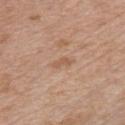{"biopsy_status": "not biopsied; imaged during a skin examination", "lesion_size": {"long_diameter_mm_approx": 2.5}, "automated_metrics": {"cielab_L": 57, "cielab_a": 19, "cielab_b": 32, "vs_skin_darker_L": 7.0, "border_irregularity_0_10": 4.5, "color_variation_0_10": 0.0, "peripheral_color_asymmetry": 0.0, "nevus_likeness_0_100": 0}, "site": "chest", "image": {"source": "total-body photography crop", "field_of_view_mm": 15}, "patient": {"sex": "male", "age_approx": 65}, "lighting": "white-light"}